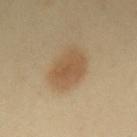biopsy status: no biopsy performed (imaged during a skin exam)
TBP lesion metrics: a mean CIELAB color near L≈55 a*≈16 b*≈35, roughly 9 lightness units darker than nearby skin, and a lesion-to-skin contrast of about 7 (normalized; higher = more distinct)
subject: female, about 40 years old
site: the mid back
image source: total-body-photography crop, ~15 mm field of view
illumination: cross-polarized illumination